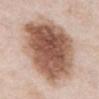anatomic site = the chest | subject = male, aged 53 to 57 | image source = total-body-photography crop, ~15 mm field of view.